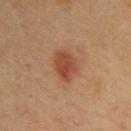Case summary:
* workup — total-body-photography surveillance lesion; no biopsy
* image-analysis metrics — an area of roughly 8.5 mm², an eccentricity of roughly 0.7, and a shape-asymmetry score of about 0.25 (0 = symmetric); about 9 CIELAB-L* units darker than the surrounding skin and a lesion-to-skin contrast of about 7.5 (normalized; higher = more distinct)
* diameter — ≈4 mm
* acquisition — ~15 mm tile from a whole-body skin photo
* body site — the back
* patient — male, in their 40s